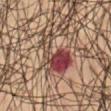Imaged during a routine full-body skin examination; the lesion was not biopsied and no histopathology is available.